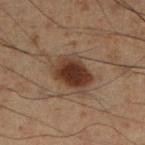The lesion was photographed on a routine skin check and not biopsied; there is no pathology result. An algorithmic analysis of the crop reported an area of roughly 13 mm², a shape eccentricity near 0.6, and a symmetry-axis asymmetry near 0.2. And it measured an automated nevus-likeness rating near 95 out of 100 and a lesion-detection confidence of about 100/100. Captured under cross-polarized illumination. A male patient roughly 50 years of age. The lesion is located on the right lower leg. Longest diameter approximately 4.5 mm. Cropped from a whole-body photographic skin survey; the tile spans about 15 mm.